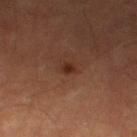Q: Was a biopsy performed?
A: imaged on a skin check; not biopsied
Q: How large is the lesion?
A: ≈1.5 mm
Q: What lighting was used for the tile?
A: cross-polarized illumination
Q: How was this image acquired?
A: ~15 mm crop, total-body skin-cancer survey
Q: Who is the patient?
A: male, aged approximately 65
Q: What is the anatomic site?
A: the right lower leg